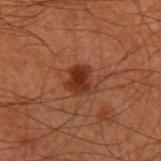Imaged during a routine full-body skin examination; the lesion was not biopsied and no histopathology is available.
Imaged with cross-polarized lighting.
A region of skin cropped from a whole-body photographic capture, roughly 15 mm wide.
Located on the left upper arm.
Automated tile analysis of the lesion measured an outline eccentricity of about 0.5 (0 = round, 1 = elongated) and two-axis asymmetry of about 0.25. It also reported a lesion color around L≈25 a*≈21 b*≈25 in CIELAB and a lesion-to-skin contrast of about 9 (normalized; higher = more distinct). The software also gave a border-irregularity rating of about 2.5/10, a within-lesion color-variation index near 3.5/10, and radial color variation of about 1. It also reported a detector confidence of about 100 out of 100 that the crop contains a lesion.
The lesion's longest dimension is about 3 mm.
A male subject, aged approximately 60.